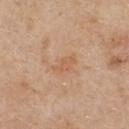Acquisition and patient details:
The lesion is on the chest. A 15 mm close-up tile from a total-body photography series done for melanoma screening. The patient is a female in their mid- to late 40s. Automated tile analysis of the lesion measured a lesion color around L≈59 a*≈21 b*≈34 in CIELAB and roughly 6 lightness units darker than nearby skin. The software also gave a border-irregularity index near 3/10, a within-lesion color-variation index near 1/10, and a peripheral color-asymmetry measure near 0.5. The tile uses white-light illumination.